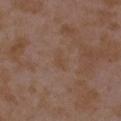workup — catalogued during a skin exam; not biopsied | anatomic site — the left upper arm | subject — female, aged approximately 35 | image source — ~15 mm crop, total-body skin-cancer survey.